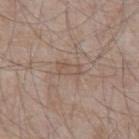<record>
  <lesion_size>
    <long_diameter_mm_approx>2.5</long_diameter_mm_approx>
  </lesion_size>
  <patient>
    <sex>male</sex>
    <age_approx>65</age_approx>
  </patient>
  <lighting>white-light</lighting>
  <site>abdomen</site>
  <image>
    <source>total-body photography crop</source>
    <field_of_view_mm>15</field_of_view_mm>
  </image>
</record>